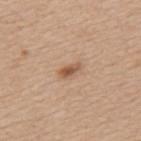Part of a total-body skin-imaging series; this lesion was reviewed on a skin check and was not flagged for biopsy. Cropped from a whole-body photographic skin survey; the tile spans about 15 mm. A female subject, aged 48 to 52. The lesion is located on the upper back.